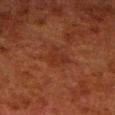{"biopsy_status": "not biopsied; imaged during a skin examination", "lesion_size": {"long_diameter_mm_approx": 3.0}, "image": {"source": "total-body photography crop", "field_of_view_mm": 15}, "site": "right lower leg", "patient": {"sex": "male", "age_approx": 80}}